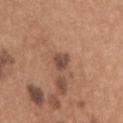workup: imaged on a skin check; not biopsied
anatomic site: the arm
lesion size: about 2.5 mm
TBP lesion metrics: a border-irregularity rating of about 3/10, internal color variation of about 2.5 on a 0–10 scale, and radial color variation of about 1
imaging modality: ~15 mm tile from a whole-body skin photo
tile lighting: white-light
subject: female, approximately 20 years of age